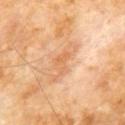Q: Was a biopsy performed?
A: catalogued during a skin exam; not biopsied
Q: How was the tile lit?
A: cross-polarized
Q: What is the anatomic site?
A: the front of the torso
Q: How was this image acquired?
A: total-body-photography crop, ~15 mm field of view
Q: What is the lesion's diameter?
A: ~3 mm (longest diameter)
Q: What are the patient's age and sex?
A: male, in their 60s